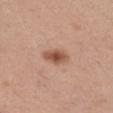• biopsy status: imaged on a skin check; not biopsied